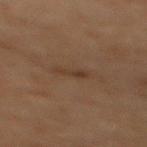Findings:
* follow-up — imaged on a skin check; not biopsied
* anatomic site — the back
* patient — male, in their 70s
* lesion diameter — ~2.5 mm (longest diameter)
* image — total-body-photography crop, ~15 mm field of view